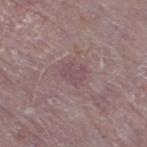The lesion was tiled from a total-body skin photograph and was not biopsied. The subject is a male about 75 years old. The lesion-visualizer software estimated a lesion-to-skin contrast of about 5 (normalized; higher = more distinct). From the right thigh. Captured under white-light illumination. A 15 mm crop from a total-body photograph taken for skin-cancer surveillance. About 3.5 mm across.